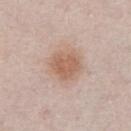Case summary:
* notes — total-body-photography surveillance lesion; no biopsy
* TBP lesion metrics — a lesion color around L≈60 a*≈19 b*≈29 in CIELAB and a lesion–skin lightness drop of about 9
* subject — female, approximately 60 years of age
* lighting — white-light illumination
* imaging modality — total-body-photography crop, ~15 mm field of view
* body site — the chest
* diameter — ~4 mm (longest diameter)